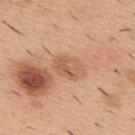Findings:
* follow-up: imaged on a skin check; not biopsied
* anatomic site: the upper back
* image source: total-body-photography crop, ~15 mm field of view
* subject: male, aged 38–42